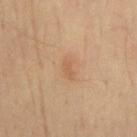workup — imaged on a skin check; not biopsied.